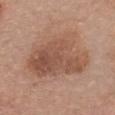Assessment: Part of a total-body skin-imaging series; this lesion was reviewed on a skin check and was not flagged for biopsy. Context: From the chest. Imaged with white-light lighting. Longest diameter approximately 8.5 mm. The subject is a female roughly 60 years of age. The total-body-photography lesion software estimated a lesion area of about 36 mm² and two-axis asymmetry of about 0.3. This image is a 15 mm lesion crop taken from a total-body photograph.